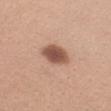Imaged during a routine full-body skin examination; the lesion was not biopsied and no histopathology is available. The tile uses white-light illumination. A female patient, aged 23–27. Located on the right forearm. A lesion tile, about 15 mm wide, cut from a 3D total-body photograph. Approximately 4 mm at its widest.